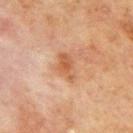Clinical impression: Captured during whole-body skin photography for melanoma surveillance; the lesion was not biopsied. Image and clinical context: The recorded lesion diameter is about 3.5 mm. Captured under cross-polarized illumination. On the mid back. Cropped from a whole-body photographic skin survey; the tile spans about 15 mm. A male patient in their 70s.